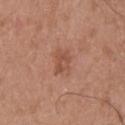<tbp_lesion>
<lesion_size>
  <long_diameter_mm_approx>3.0</long_diameter_mm_approx>
</lesion_size>
<image>
  <source>total-body photography crop</source>
  <field_of_view_mm>15</field_of_view_mm>
</image>
<automated_metrics>
  <nevus_likeness_0_100>20</nevus_likeness_0_100>
</automated_metrics>
<site>upper back</site>
<lighting>white-light</lighting>
<patient>
  <sex>male</sex>
  <age_approx>50</age_approx>
</patient>
</tbp_lesion>